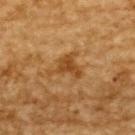No biopsy was performed on this lesion — it was imaged during a full skin examination and was not determined to be concerning.
The tile uses cross-polarized illumination.
A male patient about 85 years old or older.
Automated image analysis of the tile measured an automated nevus-likeness rating near 70 out of 100 and lesion-presence confidence of about 100/100.
Cropped from a total-body skin-imaging series; the visible field is about 15 mm.
Longest diameter approximately 4 mm.
From the upper back.